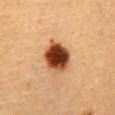Clinical impression:
The lesion was tiled from a total-body skin photograph and was not biopsied.
Acquisition and patient details:
Located on the abdomen. A region of skin cropped from a whole-body photographic capture, roughly 15 mm wide. Approximately 4 mm at its widest. A female subject about 55 years old. Captured under cross-polarized illumination.